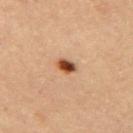This lesion was catalogued during total-body skin photography and was not selected for biopsy. This is a cross-polarized tile. Cropped from a whole-body photographic skin survey; the tile spans about 15 mm. Approximately 2.5 mm at its widest. The subject is a female approximately 35 years of age. Located on the right upper arm.